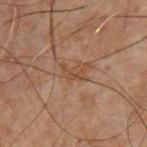  patient:
    sex: male
    age_approx: 70
  lighting: cross-polarized
  image:
    source: total-body photography crop
    field_of_view_mm: 15
  site: chest
  lesion_size:
    long_diameter_mm_approx: 2.5